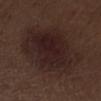Case summary:
* notes — no biopsy performed (imaged during a skin exam)
* diameter — ≈8.5 mm
* site — the right lower leg
* acquisition — ~15 mm tile from a whole-body skin photo
* tile lighting — white-light illumination
* patient — male, aged around 70